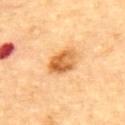workup=no biopsy performed (imaged during a skin exam) | diameter=≈3.5 mm | imaging modality=total-body-photography crop, ~15 mm field of view | anatomic site=the upper back | automated lesion analysis=a lesion color around L≈62 a*≈26 b*≈46 in CIELAB, roughly 15 lightness units darker than nearby skin, and a normalized border contrast of about 9.5 | subject=male, in their mid-80s or older.